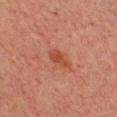Impression: The lesion was tiled from a total-body skin photograph and was not biopsied. Clinical summary: Approximately 2.5 mm at its widest. A male subject aged 28–32. A close-up tile cropped from a whole-body skin photograph, about 15 mm across. The lesion is located on the chest.